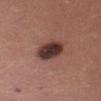The lesion was photographed on a routine skin check and not biopsied; there is no pathology result. A female subject aged 48 to 52. The lesion is on the left thigh. Captured under white-light illumination. About 4 mm across. A 15 mm close-up tile from a total-body photography series done for melanoma screening.